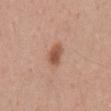Recorded during total-body skin imaging; not selected for excision or biopsy.
A male patient, aged 53–57.
On the abdomen.
Captured under white-light illumination.
A region of skin cropped from a whole-body photographic capture, roughly 15 mm wide.
Longest diameter approximately 3 mm.